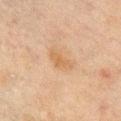| field | value |
|---|---|
| notes | catalogued during a skin exam; not biopsied |